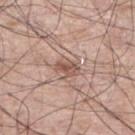The lesion was photographed on a routine skin check and not biopsied; there is no pathology result.
An algorithmic analysis of the crop reported a border-irregularity index near 3/10 and a color-variation rating of about 3.5/10. The analysis additionally found a classifier nevus-likeness of about 0/100 and a detector confidence of about 100 out of 100 that the crop contains a lesion.
This is a white-light tile.
The subject is a male in their 60s.
The lesion is on the right lower leg.
A roughly 15 mm field-of-view crop from a total-body skin photograph.
About 3 mm across.